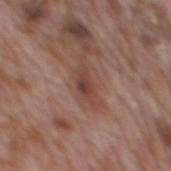Assessment:
Imaged during a routine full-body skin examination; the lesion was not biopsied and no histopathology is available.
Background:
Measured at roughly 3.5 mm in maximum diameter. On the mid back. A male subject, in their 70s. A lesion tile, about 15 mm wide, cut from a 3D total-body photograph. The tile uses white-light illumination.